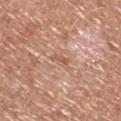follow-up = catalogued during a skin exam; not biopsied
tile lighting = white-light illumination
patient = male, aged approximately 70
image source = 15 mm crop, total-body photography
lesion size = about 2.5 mm
site = the left lower leg